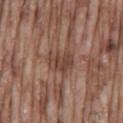  site: mid back
  patient:
    sex: male
    age_approx: 75
  image:
    source: total-body photography crop
    field_of_view_mm: 15